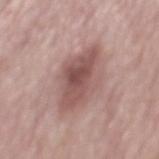biopsy status: no biopsy performed (imaged during a skin exam) | image: ~15 mm tile from a whole-body skin photo | diameter: ≈7.5 mm | subject: male, in their mid-70s | anatomic site: the mid back.